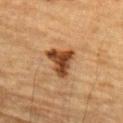Impression: Imaged during a routine full-body skin examination; the lesion was not biopsied and no histopathology is available. Acquisition and patient details: The lesion is on the left forearm. A roughly 15 mm field-of-view crop from a total-body skin photograph. A male subject, aged 83 or over.